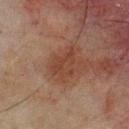Imaged during a routine full-body skin examination; the lesion was not biopsied and no histopathology is available.
A male subject, aged 63 to 67.
The lesion is located on the upper back.
A 15 mm close-up tile from a total-body photography series done for melanoma screening.
The recorded lesion diameter is about 4 mm.